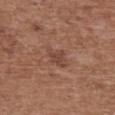Background:
A female patient, aged approximately 75. Automated image analysis of the tile measured a lesion area of about 5.5 mm². And it measured a border-irregularity index near 3.5/10, a color-variation rating of about 2/10, and peripheral color asymmetry of about 0.5. And it measured a nevus-likeness score of about 0/100 and a detector confidence of about 100 out of 100 that the crop contains a lesion. Measured at roughly 3.5 mm in maximum diameter. On the upper back. Cropped from a whole-body photographic skin survey; the tile spans about 15 mm. The tile uses white-light illumination.